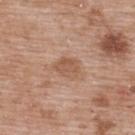Imaged during a routine full-body skin examination; the lesion was not biopsied and no histopathology is available.
Located on the upper back.
Approximately 3 mm at its widest.
The patient is a male aged 48–52.
A close-up tile cropped from a whole-body skin photograph, about 15 mm across.
The tile uses white-light illumination.